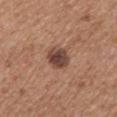Recorded during total-body skin imaging; not selected for excision or biopsy. The lesion's longest dimension is about 3 mm. Cropped from a whole-body photographic skin survey; the tile spans about 15 mm. On the mid back. The subject is a male about 65 years old. Automated image analysis of the tile measured a footprint of about 6 mm², a shape eccentricity near 0.65, and a shape-asymmetry score of about 0.15 (0 = symmetric). And it measured a mean CIELAB color near L≈43 a*≈20 b*≈25 and about 14 CIELAB-L* units darker than the surrounding skin.